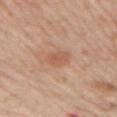Notes:
• follow-up: total-body-photography surveillance lesion; no biopsy